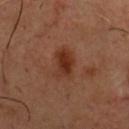The lesion was photographed on a routine skin check and not biopsied; there is no pathology result.
This image is a 15 mm lesion crop taken from a total-body photograph.
From the chest.
The subject is a male aged approximately 50.
Approximately 4 mm at its widest.
Captured under cross-polarized illumination.